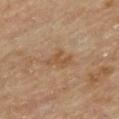biopsy_status: not biopsied; imaged during a skin examination
lesion_size:
  long_diameter_mm_approx: 3.5
patient:
  sex: male
  age_approx: 85+
site: mid back
lighting: cross-polarized
image:
  source: total-body photography crop
  field_of_view_mm: 15